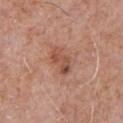The lesion was tiled from a total-body skin photograph and was not biopsied. Located on the front of the torso. About 3.5 mm across. A male subject roughly 70 years of age. An algorithmic analysis of the crop reported a lesion area of about 5.5 mm², an eccentricity of roughly 0.85, and two-axis asymmetry of about 0.35. The analysis additionally found an average lesion color of about L≈51 a*≈24 b*≈30 (CIELAB), about 10 CIELAB-L* units darker than the surrounding skin, and a normalized border contrast of about 7. It also reported a lesion-detection confidence of about 100/100. A 15 mm crop from a total-body photograph taken for skin-cancer surveillance.